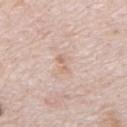Imaged during a routine full-body skin examination; the lesion was not biopsied and no histopathology is available. A male patient, about 80 years old. A roughly 15 mm field-of-view crop from a total-body skin photograph. The lesion is on the mid back. The tile uses white-light illumination. Approximately 2.5 mm at its widest. The lesion-visualizer software estimated a footprint of about 3 mm², an eccentricity of roughly 0.9, and a symmetry-axis asymmetry near 0.5. It also reported a mean CIELAB color near L≈67 a*≈17 b*≈28 and a lesion-to-skin contrast of about 5 (normalized; higher = more distinct). It also reported a border-irregularity rating of about 5.5/10 and radial color variation of about 0. The analysis additionally found a nevus-likeness score of about 0/100 and a detector confidence of about 100 out of 100 that the crop contains a lesion.